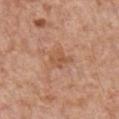automated lesion analysis: an area of roughly 4.5 mm², a shape eccentricity near 0.7, and a symmetry-axis asymmetry near 0.3; a lesion color around L≈54 a*≈23 b*≈34 in CIELAB, a lesion–skin lightness drop of about 7, and a lesion-to-skin contrast of about 5.5 (normalized; higher = more distinct); a border-irregularity rating of about 3/10 and a color-variation rating of about 2/10 | lighting: white-light | diameter: ≈3 mm | patient: male, approximately 65 years of age | body site: the chest | acquisition: 15 mm crop, total-body photography.